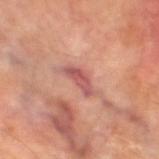Q: Is there a histopathology result?
A: catalogued during a skin exam; not biopsied
Q: Patient demographics?
A: male, aged 68 to 72
Q: Lesion location?
A: the left thigh
Q: What kind of image is this?
A: 15 mm crop, total-body photography